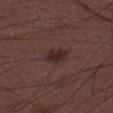workup — imaged on a skin check; not biopsied.